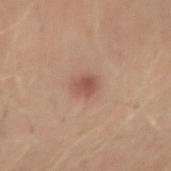image = 15 mm crop, total-body photography; patient = male, approximately 30 years of age; location = the arm.